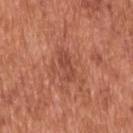- workup · imaged on a skin check; not biopsied
- patient · male, aged 63 to 67
- automated lesion analysis · border irregularity of about 2.5 on a 0–10 scale, a within-lesion color-variation index near 2.5/10, and a peripheral color-asymmetry measure near 1; a classifier nevus-likeness of about 10/100 and a lesion-detection confidence of about 100/100
- acquisition · 15 mm crop, total-body photography
- location · the upper back
- lighting · white-light illumination
- size · about 3.5 mm